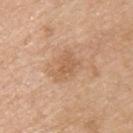No biopsy was performed on this lesion — it was imaged during a full skin examination and was not determined to be concerning. Imaged with white-light lighting. The total-body-photography lesion software estimated a footprint of about 8.5 mm² and a symmetry-axis asymmetry near 0.25. It also reported a lesion–skin lightness drop of about 7 and a normalized lesion–skin contrast near 5. It also reported a border-irregularity index near 3/10, a color-variation rating of about 2/10, and radial color variation of about 0.5. A male patient, aged 68–72. Approximately 3.5 mm at its widest. Located on the upper back. A 15 mm crop from a total-body photograph taken for skin-cancer surveillance.